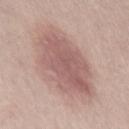Recorded during total-body skin imaging; not selected for excision or biopsy. Longest diameter approximately 10 mm. Captured under white-light illumination. The lesion-visualizer software estimated a border-irregularity rating of about 2.5/10 and a within-lesion color-variation index near 4/10. It also reported an automated nevus-likeness rating near 75 out of 100 and lesion-presence confidence of about 100/100. On the front of the torso. A female patient aged 48–52. A 15 mm close-up tile from a total-body photography series done for melanoma screening.